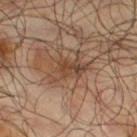  biopsy_status: not biopsied; imaged during a skin examination
  patient:
    sex: male
    age_approx: 65
  image:
    source: total-body photography crop
    field_of_view_mm: 15
  lighting: cross-polarized
  site: leg
  lesion_size:
    long_diameter_mm_approx: 5.0